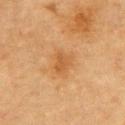Recorded during total-body skin imaging; not selected for excision or biopsy.
Automated image analysis of the tile measured a shape eccentricity near 0.6. And it measured a border-irregularity rating of about 2.5/10, internal color variation of about 2 on a 0–10 scale, and a peripheral color-asymmetry measure near 0.5. The software also gave a lesion-detection confidence of about 100/100.
A male patient aged 83 or older.
A 15 mm crop from a total-body photograph taken for skin-cancer surveillance.
The tile uses cross-polarized illumination.
From the chest.